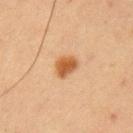Impression: Recorded during total-body skin imaging; not selected for excision or biopsy. Acquisition and patient details: The recorded lesion diameter is about 3 mm. This image is a 15 mm lesion crop taken from a total-body photograph. The subject is a male about 60 years old. From the left upper arm.